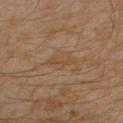Assessment:
Imaged during a routine full-body skin examination; the lesion was not biopsied and no histopathology is available.
Acquisition and patient details:
A region of skin cropped from a whole-body photographic capture, roughly 15 mm wide. The lesion-visualizer software estimated a normalized lesion–skin contrast near 5. The analysis additionally found border irregularity of about 5.5 on a 0–10 scale and a color-variation rating of about 0/10. The lesion is on the left arm. A male patient, in their 30s. Captured under cross-polarized illumination. Approximately 3 mm at its widest.